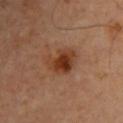Recorded during total-body skin imaging; not selected for excision or biopsy.
The lesion is located on the front of the torso.
Longest diameter approximately 4.5 mm.
A male patient in their mid- to late 50s.
A 15 mm close-up extracted from a 3D total-body photography capture.